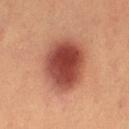Clinical impression:
No biopsy was performed on this lesion — it was imaged during a full skin examination and was not determined to be concerning.
Acquisition and patient details:
Imaged with cross-polarized lighting. A roughly 15 mm field-of-view crop from a total-body skin photograph. The lesion is located on the left thigh. About 6.5 mm across. A female subject approximately 25 years of age.